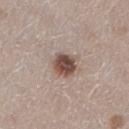<record>
  <biopsy_status>not biopsied; imaged during a skin examination</biopsy_status>
  <site>right lower leg</site>
  <patient>
    <sex>female</sex>
    <age_approx>50</age_approx>
  </patient>
  <lesion_size>
    <long_diameter_mm_approx>3.0</long_diameter_mm_approx>
  </lesion_size>
  <image>
    <source>total-body photography crop</source>
    <field_of_view_mm>15</field_of_view_mm>
  </image>
  <lighting>white-light</lighting>
  <automated_metrics>
    <cielab_L>48</cielab_L>
    <cielab_a>17</cielab_a>
    <cielab_b>23</cielab_b>
    <vs_skin_darker_L>16.0</vs_skin_darker_L>
    <border_irregularity_0_10>2.0</border_irregularity_0_10>
    <peripheral_color_asymmetry>1.5</peripheral_color_asymmetry>
  </automated_metrics>
</record>